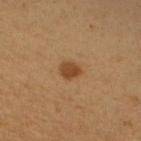| field | value |
|---|---|
| workup | imaged on a skin check; not biopsied |
| tile lighting | cross-polarized |
| anatomic site | the right upper arm |
| automated metrics | a footprint of about 4.5 mm² and an outline eccentricity of about 0.6 (0 = round, 1 = elongated); internal color variation of about 2.5 on a 0–10 scale; a classifier nevus-likeness of about 100/100 |
| lesion size | ~2.5 mm (longest diameter) |
| patient | female, in their mid- to late 50s |
| image | total-body-photography crop, ~15 mm field of view |